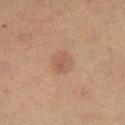A 15 mm close-up tile from a total-body photography series done for melanoma screening. On the right lower leg. This is a cross-polarized tile. Approximately 3 mm at its widest. A female subject in their mid-50s. Automated image analysis of the tile measured an outline eccentricity of about 0.45 (0 = round, 1 = elongated). And it measured a normalized border contrast of about 5. The software also gave border irregularity of about 1.5 on a 0–10 scale and a color-variation rating of about 3/10. The analysis additionally found a nevus-likeness score of about 35/100 and a lesion-detection confidence of about 100/100.